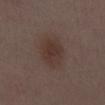| field | value |
|---|---|
| follow-up | catalogued during a skin exam; not biopsied |
| illumination | white-light illumination |
| patient | female, aged 28 to 32 |
| location | the chest |
| diameter | about 4 mm |
| acquisition | ~15 mm tile from a whole-body skin photo |
| image-analysis metrics | a footprint of about 11 mm², an outline eccentricity of about 0.6 (0 = round, 1 = elongated), and a shape-asymmetry score of about 0.15 (0 = symmetric); a mean CIELAB color near L≈34 a*≈15 b*≈20, a lesion–skin lightness drop of about 6, and a normalized lesion–skin contrast near 7; border irregularity of about 1.5 on a 0–10 scale, a color-variation rating of about 2.5/10, and peripheral color asymmetry of about 1 |